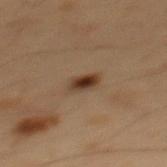Captured under cross-polarized illumination. A male patient aged 53–57. On the mid back. A close-up tile cropped from a whole-body skin photograph, about 15 mm across. The lesion-visualizer software estimated an average lesion color of about L≈29 a*≈14 b*≈24 (CIELAB) and a normalized border contrast of about 11. Longest diameter approximately 2.5 mm.